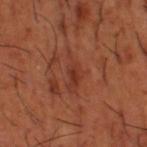Captured under cross-polarized illumination. The subject is a male in their mid- to late 60s. A close-up tile cropped from a whole-body skin photograph, about 15 mm across. The lesion is on the leg.